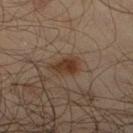| field | value |
|---|---|
| biopsy status | imaged on a skin check; not biopsied |
| image-analysis metrics | an outline eccentricity of about 0.75 (0 = round, 1 = elongated) and a symmetry-axis asymmetry near 0.25; an average lesion color of about L≈32 a*≈16 b*≈26 (CIELAB) and a lesion–skin lightness drop of about 10 |
| image | 15 mm crop, total-body photography |
| lesion diameter | about 3 mm |
| patient | male, aged 63 to 67 |
| tile lighting | cross-polarized |
| site | the right lower leg |